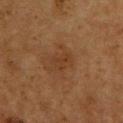Assessment:
Imaged during a routine full-body skin examination; the lesion was not biopsied and no histopathology is available.
Acquisition and patient details:
From the chest. A male subject aged 58–62. This image is a 15 mm lesion crop taken from a total-body photograph.